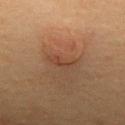Captured during whole-body skin photography for melanoma surveillance; the lesion was not biopsied.
The patient is a female in their 50s.
About 2.5 mm across.
Captured under cross-polarized illumination.
From the upper back.
A 15 mm close-up tile from a total-body photography series done for melanoma screening.